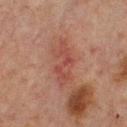| field | value |
|---|---|
| biopsy status | no biopsy performed (imaged during a skin exam) |
| anatomic site | the back |
| image | ~15 mm tile from a whole-body skin photo |
| lesion diameter | ≈6 mm |
| automated lesion analysis | a footprint of about 10 mm², an eccentricity of roughly 0.9, and a shape-asymmetry score of about 0.3 (0 = symmetric); a nevus-likeness score of about 0/100 and a detector confidence of about 100 out of 100 that the crop contains a lesion |
| illumination | cross-polarized |
| subject | male, roughly 65 years of age |